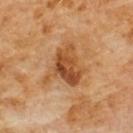– notes — catalogued during a skin exam; not biopsied
– subject — male, about 60 years old
– location — the chest
– lesion diameter — about 5.5 mm
– image — ~15 mm crop, total-body skin-cancer survey